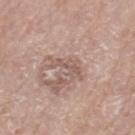The lesion was tiled from a total-body skin photograph and was not biopsied. This image is a 15 mm lesion crop taken from a total-body photograph. The patient is a female aged 73–77. On the left thigh. Automated tile analysis of the lesion measured an average lesion color of about L≈56 a*≈17 b*≈23 (CIELAB) and a lesion-to-skin contrast of about 5.5 (normalized; higher = more distinct). And it measured a border-irregularity index near 6.5/10, a within-lesion color-variation index near 2/10, and a peripheral color-asymmetry measure near 0.5. It also reported an automated nevus-likeness rating near 0 out of 100 and a detector confidence of about 95 out of 100 that the crop contains a lesion. Longest diameter approximately 3.5 mm. The tile uses white-light illumination.